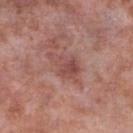Image and clinical context: This is a white-light tile. On the right lower leg. Longest diameter approximately 4 mm. Cropped from a total-body skin-imaging series; the visible field is about 15 mm. A male patient aged around 55.